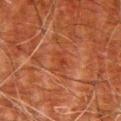Captured during whole-body skin photography for melanoma surveillance; the lesion was not biopsied. A male subject, aged approximately 80. Located on the right upper arm. A close-up tile cropped from a whole-body skin photograph, about 15 mm across. This is a cross-polarized tile.